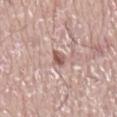Assessment: No biopsy was performed on this lesion — it was imaged during a full skin examination and was not determined to be concerning. Image and clinical context: The recorded lesion diameter is about 2.5 mm. Cropped from a whole-body photographic skin survey; the tile spans about 15 mm. A male patient aged around 75. Imaged with white-light lighting. The lesion is on the mid back.